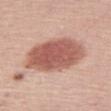workup = total-body-photography surveillance lesion; no biopsy.